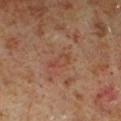| field | value |
|---|---|
| workup | no biopsy performed (imaged during a skin exam) |
| illumination | cross-polarized |
| TBP lesion metrics | an eccentricity of roughly 0.9 and a symmetry-axis asymmetry near 0.3 |
| body site | the left lower leg |
| lesion size | about 3 mm |
| subject | male, in their 60s |
| image | 15 mm crop, total-body photography |